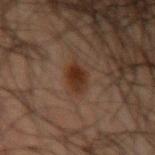• biopsy status: no biopsy performed (imaged during a skin exam)
• body site: the arm
• subject: male, about 50 years old
• imaging modality: ~15 mm tile from a whole-body skin photo
• lighting: cross-polarized illumination